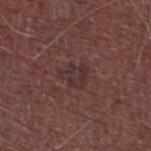notes = total-body-photography surveillance lesion; no biopsy.